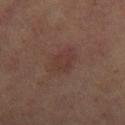location=the right thigh; lesion size=≈4.5 mm; subject=female, aged approximately 60; image source=total-body-photography crop, ~15 mm field of view; tile lighting=cross-polarized illumination.